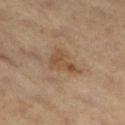biopsy status: no biopsy performed (imaged during a skin exam); subject: male, aged approximately 60; site: the leg; image source: 15 mm crop, total-body photography.